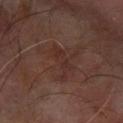Impression: Imaged during a routine full-body skin examination; the lesion was not biopsied and no histopathology is available. Acquisition and patient details: The lesion-visualizer software estimated an area of roughly 5.5 mm², a shape eccentricity near 0.95, and two-axis asymmetry of about 0.5. The analysis additionally found a mean CIELAB color near L≈27 a*≈17 b*≈20, roughly 4 lightness units darker than nearby skin, and a normalized lesion–skin contrast near 5. The analysis additionally found a border-irregularity rating of about 7/10, a color-variation rating of about 1.5/10, and radial color variation of about 0. The analysis additionally found a nevus-likeness score of about 0/100 and lesion-presence confidence of about 100/100. The lesion's longest dimension is about 5 mm. Imaged with cross-polarized lighting. A 15 mm close-up tile from a total-body photography series done for melanoma screening. The lesion is located on the left forearm. A male subject, about 65 years old.